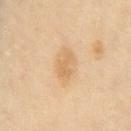Clinical impression:
The lesion was tiled from a total-body skin photograph and was not biopsied.
Acquisition and patient details:
The lesion is located on the chest. Imaged with cross-polarized lighting. Measured at roughly 3 mm in maximum diameter. A female patient about 55 years old. The lesion-visualizer software estimated radial color variation of about 0.5. The analysis additionally found an automated nevus-likeness rating near 5 out of 100 and lesion-presence confidence of about 100/100. A 15 mm close-up tile from a total-body photography series done for melanoma screening.